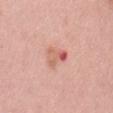Impression:
Captured during whole-body skin photography for melanoma surveillance; the lesion was not biopsied.
Context:
A female subject in their mid- to late 60s. Cropped from a whole-body photographic skin survey; the tile spans about 15 mm. From the mid back. About 3 mm across. This is a white-light tile.